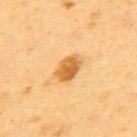Captured during whole-body skin photography for melanoma surveillance; the lesion was not biopsied.
The recorded lesion diameter is about 3.5 mm.
Cropped from a whole-body photographic skin survey; the tile spans about 15 mm.
The tile uses cross-polarized illumination.
The lesion is located on the upper back.
The subject is a male approximately 55 years of age.